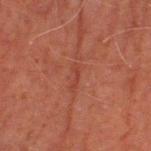biopsy_status: not biopsied; imaged during a skin examination